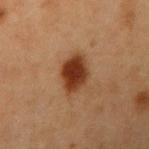<tbp_lesion>
<biopsy_status>not biopsied; imaged during a skin examination</biopsy_status>
<automated_metrics>
  <border_irregularity_0_10>1.5</border_irregularity_0_10>
  <color_variation_0_10>4.0</color_variation_0_10>
  <peripheral_color_asymmetry>1.0</peripheral_color_asymmetry>
</automated_metrics>
<patient>
  <sex>female</sex>
  <age_approx>40</age_approx>
</patient>
<image>
  <source>total-body photography crop</source>
  <field_of_view_mm>15</field_of_view_mm>
</image>
<lesion_size>
  <long_diameter_mm_approx>4.0</long_diameter_mm_approx>
</lesion_size>
<site>right upper arm</site>
<lighting>cross-polarized</lighting>
</tbp_lesion>